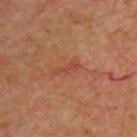This lesion was catalogued during total-body skin photography and was not selected for biopsy. From the upper back. A lesion tile, about 15 mm wide, cut from a 3D total-body photograph. A patient about 65 years old. An algorithmic analysis of the crop reported an area of roughly 3.5 mm², an outline eccentricity of about 0.9 (0 = round, 1 = elongated), and two-axis asymmetry of about 0.4. And it measured a mean CIELAB color near L≈46 a*≈27 b*≈33 and a normalized lesion–skin contrast near 4.5. It also reported radial color variation of about 0.